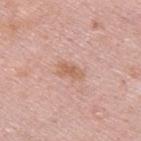Recorded during total-body skin imaging; not selected for excision or biopsy. Automated tile analysis of the lesion measured a footprint of about 4 mm², an eccentricity of roughly 0.85, and a symmetry-axis asymmetry near 0.35. The analysis additionally found a lesion color around L≈61 a*≈22 b*≈30 in CIELAB, about 8 CIELAB-L* units darker than the surrounding skin, and a normalized border contrast of about 6.5. Longest diameter approximately 3 mm. Captured under white-light illumination. A lesion tile, about 15 mm wide, cut from a 3D total-body photograph. The lesion is located on the upper back. The subject is a male in their mid-20s.